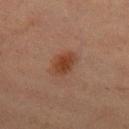Captured during whole-body skin photography for melanoma surveillance; the lesion was not biopsied.
This image is a 15 mm lesion crop taken from a total-body photograph.
On the right thigh.
A female subject, approximately 40 years of age.